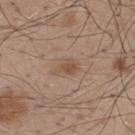Captured during whole-body skin photography for melanoma surveillance; the lesion was not biopsied. The lesion is located on the upper back. The total-body-photography lesion software estimated a nevus-likeness score of about 10/100 and a detector confidence of about 100 out of 100 that the crop contains a lesion. This image is a 15 mm lesion crop taken from a total-body photograph. Longest diameter approximately 3 mm. This is a white-light tile. A male subject aged around 50.